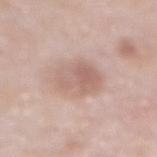The lesion was photographed on a routine skin check and not biopsied; there is no pathology result.
Approximately 4.5 mm at its widest.
Imaged with white-light lighting.
On the front of the torso.
A 15 mm close-up extracted from a 3D total-body photography capture.
A male subject aged 53 to 57.
An algorithmic analysis of the crop reported a detector confidence of about 100 out of 100 that the crop contains a lesion.